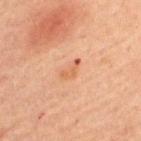Notes:
* notes: total-body-photography surveillance lesion; no biopsy
* subject: male, roughly 60 years of age
* diameter: ~3 mm (longest diameter)
* body site: the upper back
* image: 15 mm crop, total-body photography
* automated lesion analysis: a footprint of about 3 mm² and an outline eccentricity of about 0.9 (0 = round, 1 = elongated); a lesion-detection confidence of about 100/100